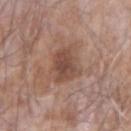This lesion was catalogued during total-body skin photography and was not selected for biopsy. From the arm. A lesion tile, about 15 mm wide, cut from a 3D total-body photograph. The tile uses white-light illumination. A male patient approximately 75 years of age. About 4.5 mm across.